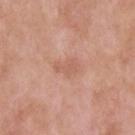The lesion was tiled from a total-body skin photograph and was not biopsied. Imaged with white-light lighting. A male patient in their mid-50s. The lesion is on the upper back. A region of skin cropped from a whole-body photographic capture, roughly 15 mm wide.